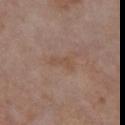The lesion was tiled from a total-body skin photograph and was not biopsied. A roughly 15 mm field-of-view crop from a total-body skin photograph. From the chest. A female patient, about 85 years old or older.